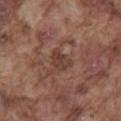follow-up: no biopsy performed (imaged during a skin exam); subject: male, about 75 years old; acquisition: 15 mm crop, total-body photography; anatomic site: the mid back; lighting: white-light.